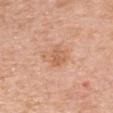Q: Was this lesion biopsied?
A: catalogued during a skin exam; not biopsied
Q: Who is the patient?
A: female, in their 50s
Q: What kind of image is this?
A: ~15 mm crop, total-body skin-cancer survey
Q: What is the lesion's diameter?
A: about 4.5 mm
Q: What lighting was used for the tile?
A: white-light
Q: Lesion location?
A: the upper back